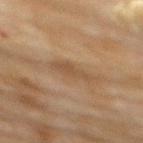No biopsy was performed on this lesion — it was imaged during a full skin examination and was not determined to be concerning. The total-body-photography lesion software estimated an average lesion color of about L≈43 a*≈15 b*≈29 (CIELAB), about 7 CIELAB-L* units darker than the surrounding skin, and a normalized lesion–skin contrast near 5.5. The software also gave a border-irregularity index near 3.5/10, a color-variation rating of about 1.5/10, and radial color variation of about 0.5. The lesion's longest dimension is about 4 mm. From the upper back. A close-up tile cropped from a whole-body skin photograph, about 15 mm across. The subject is a female roughly 80 years of age.